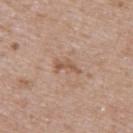Findings:
- workup — total-body-photography surveillance lesion; no biopsy
- lesion diameter — ≈3.5 mm
- image source — ~15 mm tile from a whole-body skin photo
- lighting — white-light
- location — the back
- subject — female, aged approximately 40